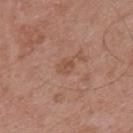Q: Is there a histopathology result?
A: catalogued during a skin exam; not biopsied
Q: What is the anatomic site?
A: the back
Q: Illumination type?
A: white-light
Q: Who is the patient?
A: male, in their mid- to late 50s
Q: Automated lesion metrics?
A: an average lesion color of about L≈51 a*≈22 b*≈28 (CIELAB) and a normalized border contrast of about 5; a border-irregularity rating of about 1.5/10, a color-variation rating of about 2.5/10, and a peripheral color-asymmetry measure near 0.5
Q: What is the imaging modality?
A: ~15 mm crop, total-body skin-cancer survey
Q: Lesion size?
A: ~2.5 mm (longest diameter)